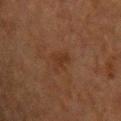Q: Is there a histopathology result?
A: no biopsy performed (imaged during a skin exam)
Q: What is the imaging modality?
A: 15 mm crop, total-body photography
Q: What is the anatomic site?
A: the upper back
Q: Patient demographics?
A: male, about 50 years old
Q: What did automated image analysis measure?
A: an area of roughly 4 mm²; a border-irregularity index near 4.5/10, internal color variation of about 1 on a 0–10 scale, and a peripheral color-asymmetry measure near 0.5; a classifier nevus-likeness of about 5/100
Q: How was the tile lit?
A: cross-polarized